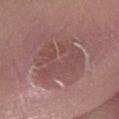No biopsy was performed on this lesion — it was imaged during a full skin examination and was not determined to be concerning.
The patient is a male aged 53 to 57.
This image is a 15 mm lesion crop taken from a total-body photograph.
On the right lower leg.